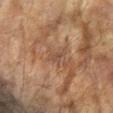Findings:
• notes: total-body-photography surveillance lesion; no biopsy
• location: the right arm
• lesion diameter: ~2.5 mm (longest diameter)
• illumination: cross-polarized illumination
• subject: female, roughly 80 years of age
• image source: total-body-photography crop, ~15 mm field of view